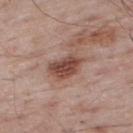{
  "biopsy_status": "not biopsied; imaged during a skin examination",
  "patient": {
    "sex": "male",
    "age_approx": 65
  },
  "site": "upper back",
  "image": {
    "source": "total-body photography crop",
    "field_of_view_mm": 15
  },
  "lesion_size": {
    "long_diameter_mm_approx": 4.5
  },
  "automated_metrics": {
    "border_irregularity_0_10": 2.5,
    "color_variation_0_10": 4.5,
    "peripheral_color_asymmetry": 1.5
  },
  "lighting": "white-light"
}